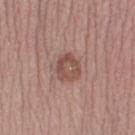notes=total-body-photography surveillance lesion; no biopsy
subject=female, roughly 40 years of age
lesion size=about 3.5 mm
body site=the right thigh
image source=15 mm crop, total-body photography
tile lighting=white-light